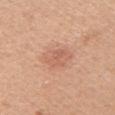The lesion was tiled from a total-body skin photograph and was not biopsied.
An algorithmic analysis of the crop reported an area of roughly 7.5 mm², a shape eccentricity near 0.7, and two-axis asymmetry of about 0.2.
The lesion's longest dimension is about 4 mm.
From the right upper arm.
A lesion tile, about 15 mm wide, cut from a 3D total-body photograph.
A female patient in their mid-40s.